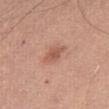• notes — imaged on a skin check; not biopsied
• lighting — white-light
• image source — total-body-photography crop, ~15 mm field of view
• lesion diameter — ≈2.5 mm
• subject — female, about 55 years old
• site — the abdomen